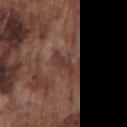Captured during whole-body skin photography for melanoma surveillance; the lesion was not biopsied. A roughly 15 mm field-of-view crop from a total-body skin photograph. A male subject in their mid-70s. Automated image analysis of the tile measured a lesion area of about 3.5 mm², an eccentricity of roughly 0.9, and two-axis asymmetry of about 0.45. It also reported internal color variation of about 1.5 on a 0–10 scale and radial color variation of about 0.5. From the chest. About 3 mm across.